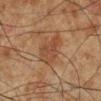image-analysis metrics=a lesion–skin lightness drop of about 6 and a normalized border contrast of about 5.5; an automated nevus-likeness rating near 5 out of 100 and a detector confidence of about 100 out of 100 that the crop contains a lesion
patient=male, aged approximately 60
imaging modality=15 mm crop, total-body photography
anatomic site=the left lower leg